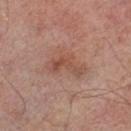* biopsy status · catalogued during a skin exam; not biopsied
* patient · male, approximately 65 years of age
* site · the left thigh
* lighting · cross-polarized illumination
* image-analysis metrics · a lesion area of about 7 mm² and a shape-asymmetry score of about 0.6 (0 = symmetric)
* acquisition · ~15 mm crop, total-body skin-cancer survey